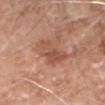{
  "biopsy_status": "not biopsied; imaged during a skin examination",
  "site": "head or neck",
  "image": {
    "source": "total-body photography crop",
    "field_of_view_mm": 15
  },
  "patient": {
    "sex": "male",
    "age_approx": 75
  },
  "automated_metrics": {
    "border_irregularity_0_10": 4.5,
    "color_variation_0_10": 4.5,
    "peripheral_color_asymmetry": 1.5,
    "nevus_likeness_0_100": 0,
    "lesion_detection_confidence_0_100": 100
  }
}